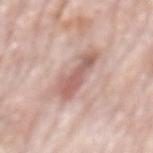Assessment:
This lesion was catalogued during total-body skin photography and was not selected for biopsy.
Background:
A male subject, in their 80s. A roughly 15 mm field-of-view crop from a total-body skin photograph. The tile uses white-light illumination. The lesion is on the mid back.